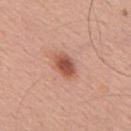follow-up: catalogued during a skin exam; not biopsied | patient: male, roughly 55 years of age | lighting: white-light | body site: the upper back | lesion size: ≈3 mm | TBP lesion metrics: border irregularity of about 1.5 on a 0–10 scale, a color-variation rating of about 4/10, and peripheral color asymmetry of about 1; a nevus-likeness score of about 95/100 | imaging modality: ~15 mm crop, total-body skin-cancer survey.